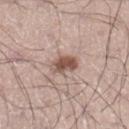follow-up — total-body-photography surveillance lesion; no biopsy
automated lesion analysis — an area of roughly 6 mm², an outline eccentricity of about 0.8 (0 = round, 1 = elongated), and two-axis asymmetry of about 0.25; border irregularity of about 2.5 on a 0–10 scale and a color-variation rating of about 4/10; a nevus-likeness score of about 95/100
acquisition — 15 mm crop, total-body photography
body site — the leg
size — about 3.5 mm
subject — male, aged 28–32